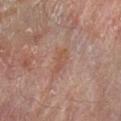Q: What lighting was used for the tile?
A: cross-polarized
Q: What are the patient's age and sex?
A: male, approximately 60 years of age
Q: How large is the lesion?
A: ≈3 mm
Q: What is the imaging modality?
A: ~15 mm crop, total-body skin-cancer survey
Q: Lesion location?
A: the arm